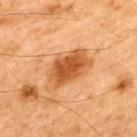Captured during whole-body skin photography for melanoma surveillance; the lesion was not biopsied.
The patient is a male aged 63–67.
The recorded lesion diameter is about 6.5 mm.
Located on the upper back.
A region of skin cropped from a whole-body photographic capture, roughly 15 mm wide.
The tile uses cross-polarized illumination.